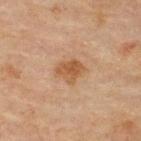The lesion was tiled from a total-body skin photograph and was not biopsied. A 15 mm crop from a total-body photograph taken for skin-cancer surveillance. The subject is a male aged 43–47. The lesion is located on the upper back.